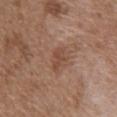The lesion is on the abdomen.
A 15 mm close-up extracted from a 3D total-body photography capture.
A male subject, approximately 75 years of age.
Measured at roughly 3 mm in maximum diameter.
This is a white-light tile.